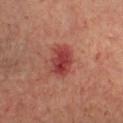Notes:
– follow-up: no biopsy performed (imaged during a skin exam)
– acquisition: total-body-photography crop, ~15 mm field of view
– anatomic site: the chest
– subject: female, in their mid- to late 40s
– lesion size: about 4 mm